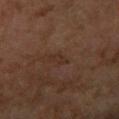<case>
<biopsy_status>not biopsied; imaged during a skin examination</biopsy_status>
<lighting>cross-polarized</lighting>
<automated_metrics>
  <area_mm2_approx>2.5</area_mm2_approx>
  <eccentricity>0.85</eccentricity>
  <shape_asymmetry>0.25</shape_asymmetry>
  <cielab_L>25</cielab_L>
  <cielab_a>15</cielab_a>
  <cielab_b>22</cielab_b>
  <vs_skin_darker_L>4.0</vs_skin_darker_L>
  <vs_skin_contrast_norm>5.0</vs_skin_contrast_norm>
  <border_irregularity_0_10>3.0</border_irregularity_0_10>
  <color_variation_0_10>0.0</color_variation_0_10>
  <nevus_likeness_0_100>0</nevus_likeness_0_100>
  <lesion_detection_confidence_0_100>100</lesion_detection_confidence_0_100>
</automated_metrics>
<patient>
  <sex>female</sex>
  <age_approx>60</age_approx>
</patient>
<image>
  <source>total-body photography crop</source>
  <field_of_view_mm>15</field_of_view_mm>
</image>
<site>arm</site>
</case>